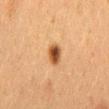The lesion was tiled from a total-body skin photograph and was not biopsied. Measured at roughly 2.5 mm in maximum diameter. On the mid back. Cropped from a total-body skin-imaging series; the visible field is about 15 mm. The tile uses cross-polarized illumination. A female patient, about 50 years old. Automated image analysis of the tile measured a lesion area of about 4.5 mm² and a shape-asymmetry score of about 0.2 (0 = symmetric). It also reported a lesion color around L≈43 a*≈20 b*≈34 in CIELAB, a lesion–skin lightness drop of about 14, and a normalized lesion–skin contrast near 10.5. And it measured a border-irregularity index near 1.5/10, a color-variation rating of about 5.5/10, and radial color variation of about 2.